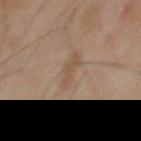Clinical impression: The lesion was photographed on a routine skin check and not biopsied; there is no pathology result. Image and clinical context: The lesion is on the chest. Cropped from a total-body skin-imaging series; the visible field is about 15 mm. Measured at roughly 3.5 mm in maximum diameter. A male patient in their mid- to late 40s.